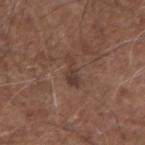Part of a total-body skin-imaging series; this lesion was reviewed on a skin check and was not flagged for biopsy.
The tile uses white-light illumination.
A male subject, in their mid- to late 70s.
A 15 mm close-up extracted from a 3D total-body photography capture.
The lesion is located on the arm.
The lesion's longest dimension is about 3.5 mm.
Automated image analysis of the tile measured a mean CIELAB color near L≈38 a*≈17 b*≈23. The software also gave a border-irregularity index near 5.5/10 and radial color variation of about 0.5. And it measured a classifier nevus-likeness of about 0/100 and a detector confidence of about 100 out of 100 that the crop contains a lesion.